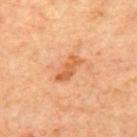<tbp_lesion>
<biopsy_status>not biopsied; imaged during a skin examination</biopsy_status>
<lighting>cross-polarized</lighting>
<site>back</site>
<lesion_size>
  <long_diameter_mm_approx>3.5</long_diameter_mm_approx>
</lesion_size>
<patient>
  <age_approx>65</age_approx>
</patient>
<image>
  <source>total-body photography crop</source>
  <field_of_view_mm>15</field_of_view_mm>
</image>
<automated_metrics>
  <area_mm2_approx>5.5</area_mm2_approx>
  <eccentricity>0.9</eccentricity>
  <shape_asymmetry>0.25</shape_asymmetry>
  <border_irregularity_0_10>3.0</border_irregularity_0_10>
  <color_variation_0_10>3.5</color_variation_0_10>
  <peripheral_color_asymmetry>1.0</peripheral_color_asymmetry>
  <nevus_likeness_0_100>5</nevus_likeness_0_100>
  <lesion_detection_confidence_0_100>100</lesion_detection_confidence_0_100>
</automated_metrics>
</tbp_lesion>